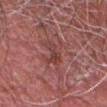Recorded during total-body skin imaging; not selected for excision or biopsy. This is a white-light tile. The patient is a male aged approximately 70. Longest diameter approximately 3.5 mm. Cropped from a whole-body photographic skin survey; the tile spans about 15 mm. The total-body-photography lesion software estimated about 8 CIELAB-L* units darker than the surrounding skin and a normalized border contrast of about 6. It also reported border irregularity of about 6 on a 0–10 scale, a within-lesion color-variation index near 1/10, and radial color variation of about 0.5. Located on the head or neck.